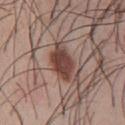biopsy status: total-body-photography surveillance lesion; no biopsy
location: the chest
imaging modality: ~15 mm tile from a whole-body skin photo
subject: male, aged around 30
lighting: white-light illumination
diameter: about 5 mm
automated metrics: a lesion area of about 9 mm², a shape eccentricity near 0.8, and a symmetry-axis asymmetry near 0.15